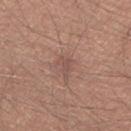Impression:
No biopsy was performed on this lesion — it was imaged during a full skin examination and was not determined to be concerning.
Acquisition and patient details:
A 15 mm crop from a total-body photograph taken for skin-cancer surveillance. Located on the back. The subject is a male about 25 years old. About 2.5 mm across.